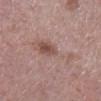Imaged during a routine full-body skin examination; the lesion was not biopsied and no histopathology is available. The lesion is located on the left lower leg. The tile uses white-light illumination. A lesion tile, about 15 mm wide, cut from a 3D total-body photograph. A female patient, about 50 years old.